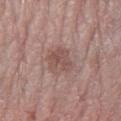biopsy status: no biopsy performed (imaged during a skin exam)
image: total-body-photography crop, ~15 mm field of view
lesion size: ≈3.5 mm
lighting: white-light
subject: female, about 65 years old
anatomic site: the left forearm
image-analysis metrics: a footprint of about 7 mm², an eccentricity of roughly 0.6, and a shape-asymmetry score of about 0.3 (0 = symmetric); a border-irregularity index near 3.5/10, a within-lesion color-variation index near 3/10, and radial color variation of about 1; a nevus-likeness score of about 10/100 and lesion-presence confidence of about 100/100